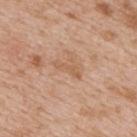{
  "biopsy_status": "not biopsied; imaged during a skin examination",
  "image": {
    "source": "total-body photography crop",
    "field_of_view_mm": 15
  },
  "site": "upper back",
  "lighting": "white-light",
  "automated_metrics": {
    "cielab_L": 58,
    "cielab_a": 21,
    "cielab_b": 34,
    "vs_skin_darker_L": 7.0,
    "vs_skin_contrast_norm": 5.5,
    "border_irregularity_0_10": 8.0,
    "color_variation_0_10": 0.0,
    "peripheral_color_asymmetry": 0.0,
    "nevus_likeness_0_100": 0,
    "lesion_detection_confidence_0_100": 100
  },
  "patient": {
    "sex": "male",
    "age_approx": 65
  }
}